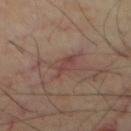Findings:
– workup · no biopsy performed (imaged during a skin exam)
– body site · the chest
– patient · in their mid- to late 60s
– TBP lesion metrics · a footprint of about 5 mm², an eccentricity of roughly 0.9, and two-axis asymmetry of about 0.45; a classifier nevus-likeness of about 0/100 and lesion-presence confidence of about 75/100
– image · ~15 mm crop, total-body skin-cancer survey
– illumination · cross-polarized
– diameter · about 4 mm